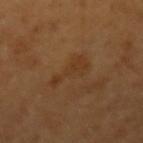Assessment:
The lesion was photographed on a routine skin check and not biopsied; there is no pathology result.
Image and clinical context:
A 15 mm close-up extracted from a 3D total-body photography capture. This is a cross-polarized tile. The recorded lesion diameter is about 4.5 mm. Automated tile analysis of the lesion measured a mean CIELAB color near L≈37 a*≈19 b*≈33 and roughly 5 lightness units darker than nearby skin. The software also gave a within-lesion color-variation index near 2/10. The lesion is on the left upper arm. The patient is a female roughly 55 years of age.